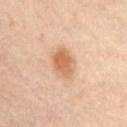No biopsy was performed on this lesion — it was imaged during a full skin examination and was not determined to be concerning.
The total-body-photography lesion software estimated an area of roughly 8.5 mm² and a symmetry-axis asymmetry near 0.2. The software also gave a mean CIELAB color near L≈66 a*≈22 b*≈38 and roughly 12 lightness units darker than nearby skin. And it measured border irregularity of about 1.5 on a 0–10 scale, a color-variation rating of about 3.5/10, and a peripheral color-asymmetry measure near 1.
On the front of the torso.
Cropped from a total-body skin-imaging series; the visible field is about 15 mm.
Longest diameter approximately 4 mm.